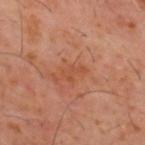follow-up = catalogued during a skin exam; not biopsied
site = the mid back
TBP lesion metrics = a shape eccentricity near 0.8 and a symmetry-axis asymmetry near 0.4; an automated nevus-likeness rating near 0 out of 100 and a lesion-detection confidence of about 100/100
tile lighting = cross-polarized
lesion size = ~3 mm (longest diameter)
image source = ~15 mm crop, total-body skin-cancer survey
subject = male, aged 58 to 62